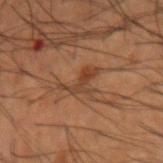Part of a total-body skin-imaging series; this lesion was reviewed on a skin check and was not flagged for biopsy. Located on the right forearm. A 15 mm close-up tile from a total-body photography series done for melanoma screening. The patient is a male aged 48 to 52.